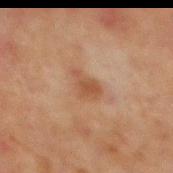The lesion was photographed on a routine skin check and not biopsied; there is no pathology result.
Cropped from a total-body skin-imaging series; the visible field is about 15 mm.
The lesion's longest dimension is about 3.5 mm.
From the front of the torso.
A male patient, aged approximately 65.